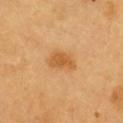biopsy_status: not biopsied; imaged during a skin examination
lighting: cross-polarized
image:
  source: total-body photography crop
  field_of_view_mm: 15
patient:
  sex: male
  age_approx: 60
lesion_size:
  long_diameter_mm_approx: 3.5
site: chest